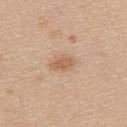{
  "biopsy_status": "not biopsied; imaged during a skin examination",
  "image": {
    "source": "total-body photography crop",
    "field_of_view_mm": 15
  },
  "lesion_size": {
    "long_diameter_mm_approx": 3.0
  },
  "automated_metrics": {
    "area_mm2_approx": 4.0,
    "eccentricity": 0.85,
    "shape_asymmetry": 0.2,
    "border_irregularity_0_10": 2.0,
    "color_variation_0_10": 2.0,
    "peripheral_color_asymmetry": 1.0,
    "nevus_likeness_0_100": 40
  },
  "lighting": "white-light",
  "site": "upper back",
  "patient": {
    "sex": "female",
    "age_approx": 45
  }
}